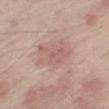{
  "image": {
    "source": "total-body photography crop",
    "field_of_view_mm": 15
  },
  "site": "right thigh",
  "patient": {
    "sex": "male",
    "age_approx": 65
  }
}